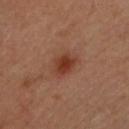Cropped from a total-body skin-imaging series; the visible field is about 15 mm.
The lesion is located on the upper back.
Automated tile analysis of the lesion measured a lesion area of about 8.5 mm², a shape eccentricity near 0.6, and a symmetry-axis asymmetry near 0.15. And it measured an average lesion color of about L≈38 a*≈23 b*≈28 (CIELAB) and a normalized lesion–skin contrast near 7.5. The software also gave a classifier nevus-likeness of about 95/100 and a lesion-detection confidence of about 100/100.
The patient is a male about 35 years old.
Measured at roughly 3.5 mm in maximum diameter.